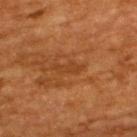follow-up = no biopsy performed (imaged during a skin exam)
imaging modality = 15 mm crop, total-body photography
site = the upper back
patient = male, aged approximately 60
illumination = cross-polarized
lesion diameter = ~3 mm (longest diameter)
TBP lesion metrics = a mean CIELAB color near L≈38 a*≈24 b*≈37 and about 6 CIELAB-L* units darker than the surrounding skin; a lesion-detection confidence of about 85/100